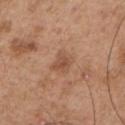<record>
<biopsy_status>not biopsied; imaged during a skin examination</biopsy_status>
<patient>
  <sex>male</sex>
  <age_approx>55</age_approx>
</patient>
<image>
  <source>total-body photography crop</source>
  <field_of_view_mm>15</field_of_view_mm>
</image>
<lighting>white-light</lighting>
<lesion_size>
  <long_diameter_mm_approx>2.5</long_diameter_mm_approx>
</lesion_size>
<site>chest</site>
</record>